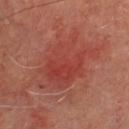<record>
  <biopsy_status>not biopsied; imaged during a skin examination</biopsy_status>
  <lighting>cross-polarized</lighting>
  <patient>
    <sex>male</sex>
    <age_approx>65</age_approx>
  </patient>
  <image>
    <source>total-body photography crop</source>
    <field_of_view_mm>15</field_of_view_mm>
  </image>
  <lesion_size>
    <long_diameter_mm_approx>8.0</long_diameter_mm_approx>
  </lesion_size>
  <site>chest</site>
</record>